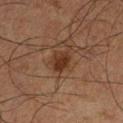• workup · total-body-photography surveillance lesion; no biopsy